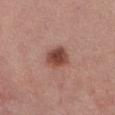Q: How large is the lesion?
A: ~3 mm (longest diameter)
Q: What is the anatomic site?
A: the front of the torso
Q: How was the tile lit?
A: white-light
Q: What are the patient's age and sex?
A: female, roughly 55 years of age
Q: What did automated image analysis measure?
A: an average lesion color of about L≈46 a*≈24 b*≈26 (CIELAB), roughly 13 lightness units darker than nearby skin, and a normalized lesion–skin contrast near 10; border irregularity of about 2 on a 0–10 scale and a within-lesion color-variation index near 4.5/10
Q: What kind of image is this?
A: 15 mm crop, total-body photography